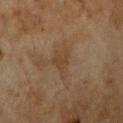Assessment:
Recorded during total-body skin imaging; not selected for excision or biopsy.
Background:
A 15 mm crop from a total-body photograph taken for skin-cancer surveillance. Measured at roughly 3.5 mm in maximum diameter. Captured under cross-polarized illumination. Located on the right upper arm. A female subject aged around 80.